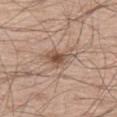The lesion is on the left thigh.
A male subject, aged 58–62.
A 15 mm close-up extracted from a 3D total-body photography capture.
The lesion's longest dimension is about 3.5 mm.